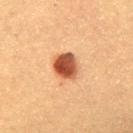A 15 mm close-up tile from a total-body photography series done for melanoma screening. The lesion-visualizer software estimated an area of roughly 8.5 mm², an outline eccentricity of about 0.5 (0 = round, 1 = elongated), and a symmetry-axis asymmetry near 0.15. The software also gave an automated nevus-likeness rating near 100 out of 100 and a lesion-detection confidence of about 100/100. A female subject, in their 60s. Measured at roughly 3.5 mm in maximum diameter. The lesion is on the upper back.